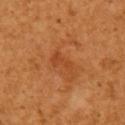<record>
<biopsy_status>not biopsied; imaged during a skin examination</biopsy_status>
<lighting>cross-polarized</lighting>
<site>right upper arm</site>
<image>
  <source>total-body photography crop</source>
  <field_of_view_mm>15</field_of_view_mm>
</image>
<lesion_size>
  <long_diameter_mm_approx>3.0</long_diameter_mm_approx>
</lesion_size>
<patient>
  <sex>female</sex>
  <age_approx>55</age_approx>
</patient>
<automated_metrics>
  <area_mm2_approx>4.0</area_mm2_approx>
  <eccentricity>0.85</eccentricity>
  <cielab_L>47</cielab_L>
  <cielab_a>30</cielab_a>
  <cielab_b>42</cielab_b>
  <vs_skin_darker_L>6.0</vs_skin_darker_L>
  <vs_skin_contrast_norm>5.0</vs_skin_contrast_norm>
  <nevus_likeness_0_100>0</nevus_likeness_0_100>
  <lesion_detection_confidence_0_100>100</lesion_detection_confidence_0_100>
</automated_metrics>
</record>